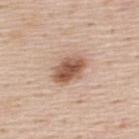  biopsy_status: not biopsied; imaged during a skin examination
  lighting: white-light
  patient:
    sex: male
    age_approx: 45
  image:
    source: total-body photography crop
    field_of_view_mm: 15
  automated_metrics:
    border_irregularity_0_10: 1.5
    color_variation_0_10: 5.5
    peripheral_color_asymmetry: 2.0
  site: upper back